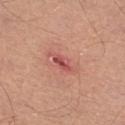{
  "biopsy_status": "not biopsied; imaged during a skin examination",
  "site": "right lower leg",
  "image": {
    "source": "total-body photography crop",
    "field_of_view_mm": 15
  },
  "patient": {
    "sex": "male",
    "age_approx": 55
  }
}